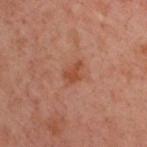imaging modality — ~15 mm crop, total-body skin-cancer survey
patient — male, in their 40s
location — the back